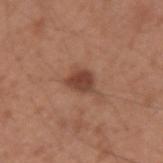notes: total-body-photography surveillance lesion; no biopsy | imaging modality: 15 mm crop, total-body photography | automated metrics: roughly 11 lightness units darker than nearby skin and a lesion-to-skin contrast of about 8.5 (normalized; higher = more distinct); border irregularity of about 3 on a 0–10 scale, a color-variation rating of about 2.5/10, and peripheral color asymmetry of about 1; a nevus-likeness score of about 70/100 and a detector confidence of about 100 out of 100 that the crop contains a lesion | diameter: ~3.5 mm (longest diameter) | lighting: white-light illumination | location: the left upper arm | subject: male, aged around 35.